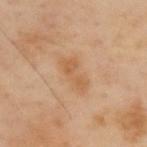{"biopsy_status": "not biopsied; imaged during a skin examination", "patient": {"sex": "male", "age_approx": 55}, "automated_metrics": {"vs_skin_contrast_norm": 6.0, "color_variation_0_10": 1.5, "nevus_likeness_0_100": 0, "lesion_detection_confidence_0_100": 100}, "image": {"source": "total-body photography crop", "field_of_view_mm": 15}, "site": "upper back"}